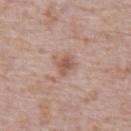follow-up = total-body-photography surveillance lesion; no biopsy | diameter = ≈2.5 mm | body site = the front of the torso | image = ~15 mm crop, total-body skin-cancer survey | subject = male, aged 68 to 72 | lighting = white-light illumination.